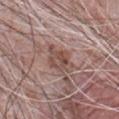Recorded during total-body skin imaging; not selected for excision or biopsy. A region of skin cropped from a whole-body photographic capture, roughly 15 mm wide. A male patient, aged approximately 70. Approximately 4.5 mm at its widest. This is a white-light tile. Located on the front of the torso.